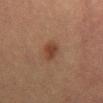Q: Was a biopsy performed?
A: imaged on a skin check; not biopsied
Q: Automated lesion metrics?
A: an area of roughly 4.5 mm²; roughly 7 lightness units darker than nearby skin and a lesion-to-skin contrast of about 7 (normalized; higher = more distinct); border irregularity of about 1.5 on a 0–10 scale, a color-variation rating of about 2/10, and a peripheral color-asymmetry measure near 0.5; a nevus-likeness score of about 90/100 and a lesion-detection confidence of about 100/100
Q: What is the imaging modality?
A: ~15 mm tile from a whole-body skin photo
Q: Illumination type?
A: cross-polarized illumination
Q: What is the lesion's diameter?
A: ≈3 mm
Q: Lesion location?
A: the abdomen
Q: Patient demographics?
A: female, roughly 55 years of age